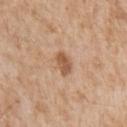Q: Is there a histopathology result?
A: imaged on a skin check; not biopsied
Q: What are the patient's age and sex?
A: male, in their mid-60s
Q: What did automated image analysis measure?
A: a lesion area of about 4.5 mm², an outline eccentricity of about 0.8 (0 = round, 1 = elongated), and a symmetry-axis asymmetry near 0.2; a border-irregularity rating of about 2/10 and peripheral color asymmetry of about 0.5; a classifier nevus-likeness of about 55/100
Q: What is the imaging modality?
A: ~15 mm crop, total-body skin-cancer survey
Q: Lesion size?
A: ~3 mm (longest diameter)
Q: Illumination type?
A: white-light
Q: Lesion location?
A: the chest